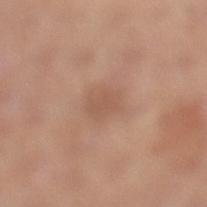  biopsy_status: not biopsied; imaged during a skin examination
  site: left lower leg
  lesion_size:
    long_diameter_mm_approx: 3.0
  patient:
    sex: female
    age_approx: 65
  image:
    source: total-body photography crop
    field_of_view_mm: 15